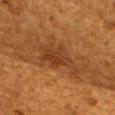Imaged during a routine full-body skin examination; the lesion was not biopsied and no histopathology is available. From the right upper arm. Approximately 7 mm at its widest. This is a cross-polarized tile. The lesion-visualizer software estimated a mean CIELAB color near L≈32 a*≈20 b*≈32, about 8 CIELAB-L* units darker than the surrounding skin, and a normalized lesion–skin contrast near 7.5. And it measured a classifier nevus-likeness of about 0/100 and lesion-presence confidence of about 75/100. The patient is a female roughly 50 years of age. A roughly 15 mm field-of-view crop from a total-body skin photograph.